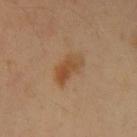subject: male, aged around 40 | lighting: cross-polarized | image source: ~15 mm tile from a whole-body skin photo | body site: the left upper arm.